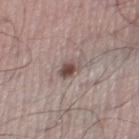follow-up — no biopsy performed (imaged during a skin exam)
TBP lesion metrics — a lesion area of about 4 mm², a shape eccentricity near 0.65, and a symmetry-axis asymmetry near 0.25; a mean CIELAB color near L≈48 a*≈16 b*≈20, a lesion–skin lightness drop of about 13, and a lesion-to-skin contrast of about 9 (normalized; higher = more distinct); a border-irregularity rating of about 2.5/10, a within-lesion color-variation index near 3.5/10, and a peripheral color-asymmetry measure near 1; a nevus-likeness score of about 95/100 and a lesion-detection confidence of about 100/100
image source — ~15 mm crop, total-body skin-cancer survey
site — the right lower leg
patient — male, aged approximately 40